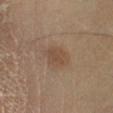workup: imaged on a skin check; not biopsied | subject: male, in their 60s | lesion size: ≈2.5 mm | site: the right lower leg | image source: total-body-photography crop, ~15 mm field of view | tile lighting: cross-polarized.